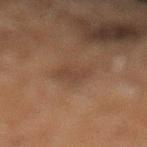biopsy_status: not biopsied; imaged during a skin examination
lesion_size:
  long_diameter_mm_approx: 3.0
site: left lower leg
automated_metrics:
  area_mm2_approx: 6.5
  eccentricity: 0.35
  shape_asymmetry: 0.2
  color_variation_0_10: 1.5
  peripheral_color_asymmetry: 0.5
  nevus_likeness_0_100: 0
  lesion_detection_confidence_0_100: 100
patient:
  sex: male
  age_approx: 75
image:
  source: total-body photography crop
  field_of_view_mm: 15
lighting: cross-polarized